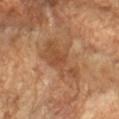| feature | finding |
|---|---|
| workup | imaged on a skin check; not biopsied |
| anatomic site | the left forearm |
| lesion diameter | about 7 mm |
| subject | female, approximately 70 years of age |
| illumination | cross-polarized illumination |
| image source | 15 mm crop, total-body photography |